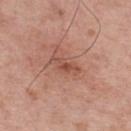This lesion was catalogued during total-body skin photography and was not selected for biopsy. A male patient, roughly 65 years of age. The lesion is located on the upper back. A 15 mm close-up extracted from a 3D total-body photography capture.